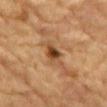Recorded during total-body skin imaging; not selected for excision or biopsy.
A 15 mm crop from a total-body photograph taken for skin-cancer surveillance.
The patient is a male approximately 85 years of age or older.
Longest diameter approximately 4 mm.
The lesion is located on the abdomen.
The tile uses cross-polarized illumination.